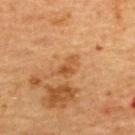The lesion was tiled from a total-body skin photograph and was not biopsied. A female subject, aged 68 to 72. The lesion is on the upper back. Automated tile analysis of the lesion measured a detector confidence of about 100 out of 100 that the crop contains a lesion. A 15 mm close-up tile from a total-body photography series done for melanoma screening. Approximately 2.5 mm at its widest.